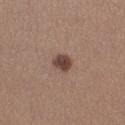{
  "biopsy_status": "not biopsied; imaged during a skin examination",
  "lighting": "white-light",
  "image": {
    "source": "total-body photography crop",
    "field_of_view_mm": 15
  },
  "site": "left lower leg",
  "patient": {
    "sex": "female",
    "age_approx": 35
  },
  "lesion_size": {
    "long_diameter_mm_approx": 2.0
  }
}